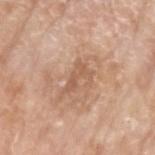Notes:
– notes · catalogued during a skin exam; not biopsied
– acquisition · total-body-photography crop, ~15 mm field of view
– subject · female, approximately 75 years of age
– tile lighting · white-light
– body site · the left upper arm
– lesion diameter · about 3.5 mm